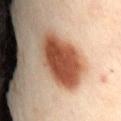Notes:
* biopsy status: catalogued during a skin exam; not biopsied
* lesion size: ~7 mm (longest diameter)
* tile lighting: cross-polarized illumination
* anatomic site: the lower back
* image: 15 mm crop, total-body photography
* automated metrics: an average lesion color of about L≈51 a*≈26 b*≈34 (CIELAB) and a lesion-to-skin contrast of about 13 (normalized; higher = more distinct); border irregularity of about 2 on a 0–10 scale, a color-variation rating of about 5/10, and a peripheral color-asymmetry measure near 1.5; a nevus-likeness score of about 100/100 and lesion-presence confidence of about 100/100
* patient: female, aged 53 to 57